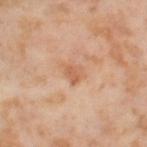follow-up: imaged on a skin check; not biopsied | imaging modality: ~15 mm crop, total-body skin-cancer survey | site: the leg | subject: female, roughly 55 years of age.